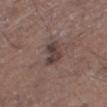<tbp_lesion>
  <biopsy_status>not biopsied; imaged during a skin examination</biopsy_status>
  <automated_metrics>
    <area_mm2_approx>6.0</area_mm2_approx>
    <eccentricity>0.75</eccentricity>
    <shape_asymmetry>0.35</shape_asymmetry>
    <cielab_L>39</cielab_L>
    <cielab_a>15</cielab_a>
    <cielab_b>17</cielab_b>
    <vs_skin_darker_L>10.0</vs_skin_darker_L>
    <vs_skin_contrast_norm>8.5</vs_skin_contrast_norm>
  </automated_metrics>
  <image>
    <source>total-body photography crop</source>
    <field_of_view_mm>15</field_of_view_mm>
  </image>
  <patient>
    <sex>male</sex>
    <age_approx>70</age_approx>
  </patient>
  <lighting>white-light</lighting>
  <site>right thigh</site>
</tbp_lesion>